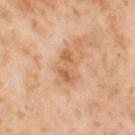Clinical impression:
Captured during whole-body skin photography for melanoma surveillance; the lesion was not biopsied.
Context:
Automated image analysis of the tile measured an eccentricity of roughly 0.85 and a symmetry-axis asymmetry near 0.3. And it measured an average lesion color of about L≈62 a*≈23 b*≈38 (CIELAB), a lesion–skin lightness drop of about 9, and a lesion-to-skin contrast of about 6.5 (normalized; higher = more distinct). The software also gave a border-irregularity index near 4/10, a within-lesion color-variation index near 3.5/10, and radial color variation of about 1. On the left thigh. Captured under cross-polarized illumination. A close-up tile cropped from a whole-body skin photograph, about 15 mm across. A female subject, in their mid-50s. Longest diameter approximately 4 mm.